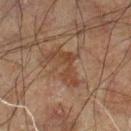notes: total-body-photography surveillance lesion; no biopsy
location: the left leg
imaging modality: ~15 mm crop, total-body skin-cancer survey
patient: male, about 60 years old